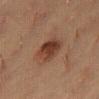<lesion>
  <biopsy_status>not biopsied; imaged during a skin examination</biopsy_status>
  <site>right thigh</site>
  <patient>
    <sex>female</sex>
    <age_approx>20</age_approx>
  </patient>
  <lighting>cross-polarized</lighting>
  <lesion_size>
    <long_diameter_mm_approx>3.5</long_diameter_mm_approx>
  </lesion_size>
  <image>
    <source>total-body photography crop</source>
    <field_of_view_mm>15</field_of_view_mm>
  </image>
</lesion>